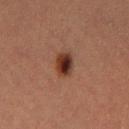Q: Is there a histopathology result?
A: no biopsy performed (imaged during a skin exam)
Q: What is the imaging modality?
A: ~15 mm crop, total-body skin-cancer survey
Q: What is the anatomic site?
A: the left thigh
Q: What are the patient's age and sex?
A: female, aged approximately 40
Q: Automated lesion metrics?
A: a lesion area of about 5.5 mm², an eccentricity of roughly 0.7, and a symmetry-axis asymmetry near 0.1; a border-irregularity index near 1/10, a color-variation rating of about 6/10, and peripheral color asymmetry of about 2; a lesion-detection confidence of about 100/100